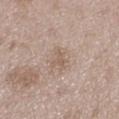Findings:
• biopsy status: no biopsy performed (imaged during a skin exam)
• body site: the left thigh
• image: ~15 mm tile from a whole-body skin photo
• subject: male, about 50 years old
• lighting: white-light illumination